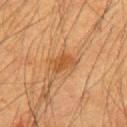Q: Was this lesion biopsied?
A: total-body-photography surveillance lesion; no biopsy
Q: What is the imaging modality?
A: ~15 mm tile from a whole-body skin photo
Q: How large is the lesion?
A: about 2.5 mm
Q: Illumination type?
A: cross-polarized illumination
Q: Patient demographics?
A: male, in their mid- to late 30s
Q: Automated lesion metrics?
A: a nevus-likeness score of about 65/100 and lesion-presence confidence of about 100/100
Q: What is the anatomic site?
A: the back